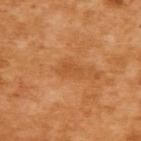Part of a total-body skin-imaging series; this lesion was reviewed on a skin check and was not flagged for biopsy. The subject is a female roughly 55 years of age. A roughly 15 mm field-of-view crop from a total-body skin photograph. The lesion is located on the upper back.